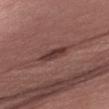Q: Was this lesion biopsied?
A: total-body-photography surveillance lesion; no biopsy
Q: What is the anatomic site?
A: the right upper arm
Q: What is the imaging modality?
A: ~15 mm crop, total-body skin-cancer survey
Q: Patient demographics?
A: female, approximately 50 years of age